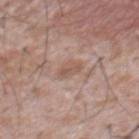The lesion was photographed on a routine skin check and not biopsied; there is no pathology result.
The lesion is on the chest.
A male patient, aged around 45.
A close-up tile cropped from a whole-body skin photograph, about 15 mm across.
Captured under white-light illumination.